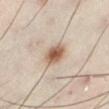Captured during whole-body skin photography for melanoma surveillance; the lesion was not biopsied.
Captured under cross-polarized illumination.
The lesion-visualizer software estimated an area of roughly 7 mm². It also reported a lesion color around L≈47 a*≈14 b*≈24 in CIELAB and a normalized lesion–skin contrast near 9.5. The software also gave a border-irregularity index near 1.5/10.
From the left lower leg.
The recorded lesion diameter is about 3.5 mm.
The subject is a male aged around 50.
A close-up tile cropped from a whole-body skin photograph, about 15 mm across.